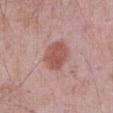Captured during whole-body skin photography for melanoma surveillance; the lesion was not biopsied.
This is a white-light tile.
Cropped from a total-body skin-imaging series; the visible field is about 15 mm.
The subject is a male aged approximately 70.
The lesion is on the abdomen.
Automated tile analysis of the lesion measured a mean CIELAB color near L≈54 a*≈24 b*≈25, roughly 10 lightness units darker than nearby skin, and a normalized border contrast of about 7.5. It also reported an automated nevus-likeness rating near 95 out of 100.